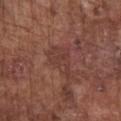notes: no biopsy performed (imaged during a skin exam); lesion diameter: ≈4.5 mm; body site: the chest; image: 15 mm crop, total-body photography; subject: male, approximately 75 years of age; lighting: white-light illumination.